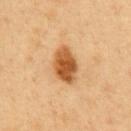The lesion was tiled from a total-body skin photograph and was not biopsied. A region of skin cropped from a whole-body photographic capture, roughly 15 mm wide. On the front of the torso. An algorithmic analysis of the crop reported an area of roughly 11 mm² and a shape eccentricity near 0.75. And it measured border irregularity of about 1.5 on a 0–10 scale and a color-variation rating of about 5/10. And it measured lesion-presence confidence of about 100/100. A male subject, roughly 50 years of age. Longest diameter approximately 4.5 mm.